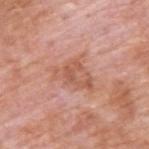<lesion>
  <biopsy_status>not biopsied; imaged during a skin examination</biopsy_status>
  <patient>
    <sex>male</sex>
    <age_approx>60</age_approx>
  </patient>
  <lighting>white-light</lighting>
  <lesion_size>
    <long_diameter_mm_approx>5.0</long_diameter_mm_approx>
  </lesion_size>
  <site>back</site>
  <image>
    <source>total-body photography crop</source>
    <field_of_view_mm>15</field_of_view_mm>
  </image>
  <automated_metrics>
    <cielab_L>58</cielab_L>
    <cielab_a>24</cielab_a>
    <cielab_b>31</cielab_b>
    <vs_skin_darker_L>8.0</vs_skin_darker_L>
    <vs_skin_contrast_norm>6.0</vs_skin_contrast_norm>
  </automated_metrics>
</lesion>